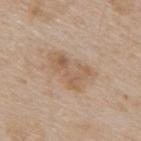Acquisition and patient details: A male subject about 65 years old. A 15 mm crop from a total-body photograph taken for skin-cancer surveillance. The lesion is on the upper back.